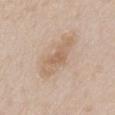No biopsy was performed on this lesion — it was imaged during a full skin examination and was not determined to be concerning.
A 15 mm close-up extracted from a 3D total-body photography capture.
A male subject, aged approximately 65.
From the abdomen.
Automated image analysis of the tile measured an automated nevus-likeness rating near 0 out of 100 and a detector confidence of about 100 out of 100 that the crop contains a lesion.
The lesion's longest dimension is about 6 mm.